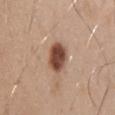Part of a total-body skin-imaging series; this lesion was reviewed on a skin check and was not flagged for biopsy. A male subject aged around 30. This image is a 15 mm lesion crop taken from a total-body photograph. Located on the chest.